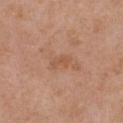A lesion tile, about 15 mm wide, cut from a 3D total-body photograph.
Automated image analysis of the tile measured a footprint of about 3 mm², a shape eccentricity near 0.85, and a shape-asymmetry score of about 0.35 (0 = symmetric). The analysis additionally found a mean CIELAB color near L≈54 a*≈22 b*≈33, about 6 CIELAB-L* units darker than the surrounding skin, and a lesion-to-skin contrast of about 5 (normalized; higher = more distinct). The software also gave border irregularity of about 4 on a 0–10 scale, internal color variation of about 0 on a 0–10 scale, and peripheral color asymmetry of about 0. And it measured a nevus-likeness score of about 0/100 and lesion-presence confidence of about 100/100.
The tile uses white-light illumination.
About 2.5 mm across.
A female patient roughly 40 years of age.
From the chest.